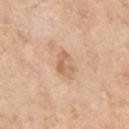notes = imaged on a skin check; not biopsied | anatomic site = the left upper arm | size = about 3 mm | subject = female, in their mid- to late 70s | image-analysis metrics = a shape eccentricity near 0.8; a lesion color around L≈64 a*≈19 b*≈36 in CIELAB, a lesion–skin lightness drop of about 9, and a normalized lesion–skin contrast near 6 | image = ~15 mm crop, total-body skin-cancer survey.